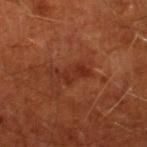Clinical impression:
Imaged during a routine full-body skin examination; the lesion was not biopsied and no histopathology is available.
Background:
A male patient aged around 65. A 15 mm close-up tile from a total-body photography series done for melanoma screening. Measured at roughly 4 mm in maximum diameter. From the right lower leg.